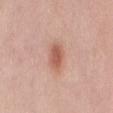No biopsy was performed on this lesion — it was imaged during a full skin examination and was not determined to be concerning. A 15 mm close-up extracted from a 3D total-body photography capture. About 3.5 mm across. A female subject, in their 60s. Located on the mid back.